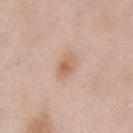follow-up = catalogued during a skin exam; not biopsied
acquisition = 15 mm crop, total-body photography
anatomic site = the chest
illumination = white-light
patient = male, roughly 55 years of age